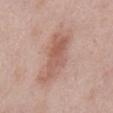Notes:
• notes · total-body-photography surveillance lesion; no biopsy
• diameter · about 6.5 mm
• acquisition · 15 mm crop, total-body photography
• subject · male, in their mid- to late 50s
• body site · the abdomen
• lighting · white-light